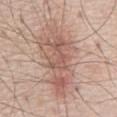• workup · imaged on a skin check; not biopsied
• lesion size · about 10.5 mm
• body site · the front of the torso
• lighting · white-light
• patient · male, about 70 years old
• acquisition · 15 mm crop, total-body photography